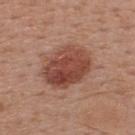Q: Was this lesion biopsied?
A: catalogued during a skin exam; not biopsied
Q: Lesion location?
A: the upper back
Q: What kind of image is this?
A: 15 mm crop, total-body photography
Q: Automated lesion metrics?
A: a mean CIELAB color near L≈46 a*≈24 b*≈27 and roughly 12 lightness units darker than nearby skin; a border-irregularity index near 1.5/10, a within-lesion color-variation index near 6/10, and peripheral color asymmetry of about 2
Q: Patient demographics?
A: male, aged around 55
Q: How was the tile lit?
A: white-light